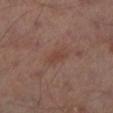The lesion was photographed on a routine skin check and not biopsied; there is no pathology result. The lesion is located on the left thigh. A male subject aged approximately 65. The lesion's longest dimension is about 2.5 mm. Cropped from a total-body skin-imaging series; the visible field is about 15 mm. Imaged with cross-polarized lighting.